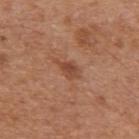The lesion was tiled from a total-body skin photograph and was not biopsied. This image is a 15 mm lesion crop taken from a total-body photograph. The subject is a male aged around 65. From the left upper arm. The recorded lesion diameter is about 3.5 mm. Imaged with white-light lighting.